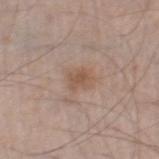Captured during whole-body skin photography for melanoma surveillance; the lesion was not biopsied. A male subject aged around 60. The lesion is on the right thigh. The lesion's longest dimension is about 2.5 mm. A 15 mm close-up tile from a total-body photography series done for melanoma screening.